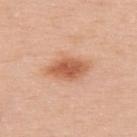Imaged during a routine full-body skin examination; the lesion was not biopsied and no histopathology is available.